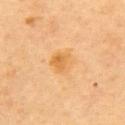Q: Was this lesion biopsied?
A: no biopsy performed (imaged during a skin exam)
Q: How large is the lesion?
A: about 3 mm
Q: What kind of image is this?
A: 15 mm crop, total-body photography
Q: What are the patient's age and sex?
A: female, about 60 years old
Q: Where on the body is the lesion?
A: the back
Q: Illumination type?
A: cross-polarized